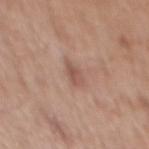Q: Is there a histopathology result?
A: imaged on a skin check; not biopsied
Q: Patient demographics?
A: male, about 70 years old
Q: How was the tile lit?
A: white-light illumination
Q: Lesion location?
A: the back
Q: How was this image acquired?
A: ~15 mm crop, total-body skin-cancer survey